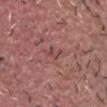The patient is a male in their 50s.
Measured at roughly 2.5 mm in maximum diameter.
Captured under white-light illumination.
On the front of the torso.
Cropped from a whole-body photographic skin survey; the tile spans about 15 mm.